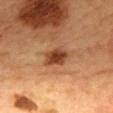* workup — no biopsy performed (imaged during a skin exam)
* anatomic site — the back
* image — ~15 mm crop, total-body skin-cancer survey
* patient — female, about 60 years old